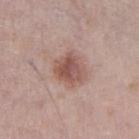Imaged during a routine full-body skin examination; the lesion was not biopsied and no histopathology is available.
An algorithmic analysis of the crop reported a footprint of about 9 mm² and two-axis asymmetry of about 0.25. The software also gave a lesion color around L≈53 a*≈21 b*≈24 in CIELAB, a lesion–skin lightness drop of about 11, and a lesion-to-skin contrast of about 8 (normalized; higher = more distinct). The software also gave a border-irregularity index near 2.5/10, a within-lesion color-variation index near 5.5/10, and radial color variation of about 1.5.
The recorded lesion diameter is about 3.5 mm.
This image is a 15 mm lesion crop taken from a total-body photograph.
A male subject, aged 73 to 77.
From the right thigh.